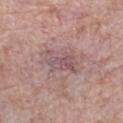This lesion was catalogued during total-body skin photography and was not selected for biopsy.